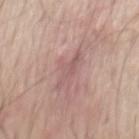Part of a total-body skin-imaging series; this lesion was reviewed on a skin check and was not flagged for biopsy. This is a white-light tile. Located on the mid back. This image is a 15 mm lesion crop taken from a total-body photograph. Measured at roughly 4 mm in maximum diameter. A male patient, in their mid-60s.